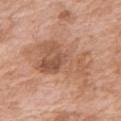Impression: Imaged during a routine full-body skin examination; the lesion was not biopsied and no histopathology is available. Background: This image is a 15 mm lesion crop taken from a total-body photograph. The patient is a female aged around 75. On the right upper arm.